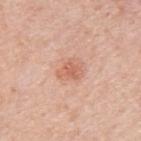Impression:
No biopsy was performed on this lesion — it was imaged during a full skin examination and was not determined to be concerning.
Acquisition and patient details:
The lesion is on the upper back. Measured at roughly 2.5 mm in maximum diameter. A 15 mm crop from a total-body photograph taken for skin-cancer surveillance. A female patient in their mid-50s. The tile uses white-light illumination.